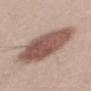Part of a total-body skin-imaging series; this lesion was reviewed on a skin check and was not flagged for biopsy.
A male patient, aged 23 to 27.
The tile uses white-light illumination.
A lesion tile, about 15 mm wide, cut from a 3D total-body photograph.
From the upper back.
Automated tile analysis of the lesion measured an area of roughly 30 mm², an outline eccentricity of about 0.85 (0 = round, 1 = elongated), and a shape-asymmetry score of about 0.1 (0 = symmetric).
Longest diameter approximately 8.5 mm.